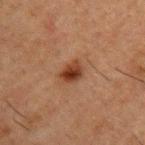biopsy status: catalogued during a skin exam; not biopsied
illumination: cross-polarized
subject: male, in their 50s
lesion diameter: about 2.5 mm
image: ~15 mm crop, total-body skin-cancer survey
image-analysis metrics: an area of roughly 4.5 mm² and a symmetry-axis asymmetry near 0.25; an automated nevus-likeness rating near 100 out of 100
anatomic site: the arm